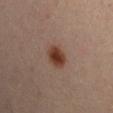lesion diameter — about 2.5 mm
patient — male, aged 33–37
acquisition — total-body-photography crop, ~15 mm field of view
body site — the arm